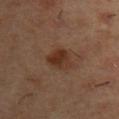{"patient": {"sex": "male", "age_approx": 55}, "lesion_size": {"long_diameter_mm_approx": 3.5}, "site": "left upper arm", "image": {"source": "total-body photography crop", "field_of_view_mm": 15}}